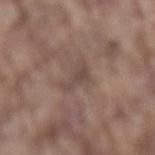Assessment: Captured during whole-body skin photography for melanoma surveillance; the lesion was not biopsied. Clinical summary: On the lower back. An algorithmic analysis of the crop reported an area of roughly 5.5 mm², a shape eccentricity near 0.45, and two-axis asymmetry of about 0.5. The analysis additionally found a mean CIELAB color near L≈46 a*≈14 b*≈21 and roughly 6 lightness units darker than nearby skin. A lesion tile, about 15 mm wide, cut from a 3D total-body photograph. Imaged with white-light lighting. The subject is a male in their mid-70s.